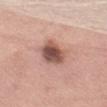Q: Was this lesion biopsied?
A: total-body-photography surveillance lesion; no biopsy
Q: What is the lesion's diameter?
A: ~4 mm (longest diameter)
Q: How was the tile lit?
A: white-light
Q: What are the patient's age and sex?
A: female, in their mid- to late 50s
Q: Lesion location?
A: the left thigh
Q: How was this image acquired?
A: ~15 mm crop, total-body skin-cancer survey
Q: Automated lesion metrics?
A: a footprint of about 10 mm² and a shape-asymmetry score of about 0.2 (0 = symmetric); a mean CIELAB color near L≈53 a*≈22 b*≈25 and a lesion–skin lightness drop of about 15; a border-irregularity rating of about 2.5/10 and a color-variation rating of about 6.5/10; a classifier nevus-likeness of about 85/100